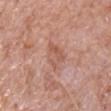{"biopsy_status": "not biopsied; imaged during a skin examination", "lighting": "white-light", "site": "right upper arm", "image": {"source": "total-body photography crop", "field_of_view_mm": 15}, "lesion_size": {"long_diameter_mm_approx": 3.0}, "patient": {"sex": "male", "age_approx": 70}}